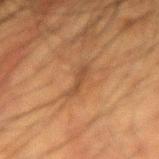follow-up: imaged on a skin check; not biopsied | TBP lesion metrics: a lesion area of about 3 mm², an outline eccentricity of about 0.95 (0 = round, 1 = elongated), and two-axis asymmetry of about 0.4 | diameter: ≈3.5 mm | subject: male, roughly 60 years of age | image source: 15 mm crop, total-body photography | location: the arm.